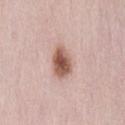workup: imaged on a skin check; not biopsied | image: ~15 mm tile from a whole-body skin photo | lesion diameter: ≈3.5 mm | location: the back | illumination: white-light | patient: female, aged around 65 | image-analysis metrics: a mean CIELAB color near L≈56 a*≈21 b*≈26, about 16 CIELAB-L* units darker than the surrounding skin, and a lesion-to-skin contrast of about 10.5 (normalized; higher = more distinct); a classifier nevus-likeness of about 100/100 and a lesion-detection confidence of about 100/100.